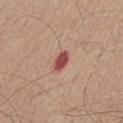Case summary:
• image source — ~15 mm crop, total-body skin-cancer survey
• body site — the abdomen
• diameter — ≈3 mm
• patient — male, aged 73 to 77